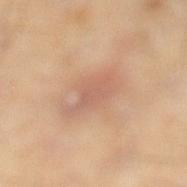| feature | finding |
|---|---|
| workup | no biopsy performed (imaged during a skin exam) |
| automated lesion analysis | an area of roughly 11 mm² and an outline eccentricity of about 0.75 (0 = round, 1 = elongated); border irregularity of about 3 on a 0–10 scale, a color-variation rating of about 3/10, and radial color variation of about 1 |
| anatomic site | the left lower leg |
| size | about 4.5 mm |
| patient | male, aged 43 to 47 |
| illumination | cross-polarized illumination |
| imaging modality | 15 mm crop, total-body photography |